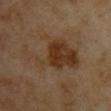Assessment: Imaged during a routine full-body skin examination; the lesion was not biopsied and no histopathology is available. Image and clinical context: A female subject, aged 58 to 62. A close-up tile cropped from a whole-body skin photograph, about 15 mm across. The tile uses cross-polarized illumination. On the arm.